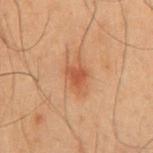{
  "lighting": "cross-polarized",
  "automated_metrics": {
    "shape_asymmetry": 0.4,
    "cielab_L": 40,
    "cielab_a": 21,
    "cielab_b": 29,
    "vs_skin_darker_L": 8.0,
    "peripheral_color_asymmetry": 1.0,
    "nevus_likeness_0_100": 75,
    "lesion_detection_confidence_0_100": 100
  },
  "patient": {
    "sex": "male",
    "age_approx": 50
  },
  "site": "front of the torso",
  "lesion_size": {
    "long_diameter_mm_approx": 2.5
  },
  "image": {
    "source": "total-body photography crop",
    "field_of_view_mm": 15
  }
}